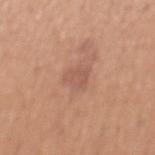Impression: The lesion was photographed on a routine skin check and not biopsied; there is no pathology result. Acquisition and patient details: An algorithmic analysis of the crop reported an outline eccentricity of about 0.45 (0 = round, 1 = elongated) and a symmetry-axis asymmetry near 0.4. The software also gave a mean CIELAB color near L≈55 a*≈22 b*≈29, roughly 7 lightness units darker than nearby skin, and a normalized lesion–skin contrast near 5. Approximately 2.5 mm at its widest. This is a white-light tile. Cropped from a total-body skin-imaging series; the visible field is about 15 mm. On the back. A male patient about 55 years old.